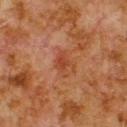<lesion>
  <biopsy_status>not biopsied; imaged during a skin examination</biopsy_status>
  <site>upper back</site>
  <image>
    <source>total-body photography crop</source>
    <field_of_view_mm>15</field_of_view_mm>
  </image>
  <patient>
    <sex>male</sex>
    <age_approx>80</age_approx>
  </patient>
</lesion>